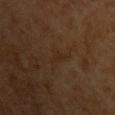workup = imaged on a skin check; not biopsied
site = the left upper arm
image source = ~15 mm crop, total-body skin-cancer survey
patient = male, aged around 60
lesion diameter = ~3 mm (longest diameter)